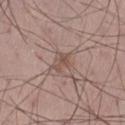Part of a total-body skin-imaging series; this lesion was reviewed on a skin check and was not flagged for biopsy.
The lesion's longest dimension is about 3 mm.
A male patient, roughly 60 years of age.
From the left thigh.
Captured under white-light illumination.
This image is a 15 mm lesion crop taken from a total-body photograph.